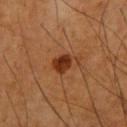{
  "biopsy_status": "not biopsied; imaged during a skin examination",
  "site": "left upper arm",
  "patient": {
    "sex": "male",
    "age_approx": 70
  },
  "lesion_size": {
    "long_diameter_mm_approx": 3.5
  },
  "lighting": "cross-polarized",
  "image": {
    "source": "total-body photography crop",
    "field_of_view_mm": 15
  },
  "automated_metrics": {
    "area_mm2_approx": 5.5,
    "shape_asymmetry": 0.3,
    "cielab_L": 34,
    "cielab_a": 25,
    "cielab_b": 34,
    "vs_skin_darker_L": 12.0,
    "vs_skin_contrast_norm": 10.5,
    "nevus_likeness_0_100": 95,
    "lesion_detection_confidence_0_100": 100
  }
}